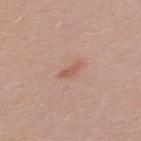<lesion>
  <biopsy_status>not biopsied; imaged during a skin examination</biopsy_status>
  <automated_metrics>
    <color_variation_0_10>0.0</color_variation_0_10>
    <peripheral_color_asymmetry>0.0</peripheral_color_asymmetry>
    <nevus_likeness_0_100>20</nevus_likeness_0_100>
    <lesion_detection_confidence_0_100>100</lesion_detection_confidence_0_100>
  </automated_metrics>
  <lesion_size>
    <long_diameter_mm_approx>2.5</long_diameter_mm_approx>
  </lesion_size>
  <site>upper back</site>
  <lighting>white-light</lighting>
  <image>
    <source>total-body photography crop</source>
    <field_of_view_mm>15</field_of_view_mm>
  </image>
  <patient>
    <sex>male</sex>
    <age_approx>40</age_approx>
  </patient>
</lesion>